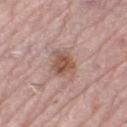Impression: The lesion was photographed on a routine skin check and not biopsied; there is no pathology result.